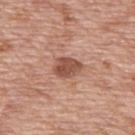Findings:
- workup — catalogued during a skin exam; not biopsied
- site — the back
- imaging modality — ~15 mm crop, total-body skin-cancer survey
- patient — male, approximately 70 years of age